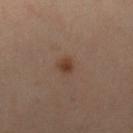This is a cross-polarized tile. About 2 mm across. A female subject aged approximately 40. A close-up tile cropped from a whole-body skin photograph, about 15 mm across. On the right lower leg. The lesion-visualizer software estimated a border-irregularity rating of about 2/10 and radial color variation of about 0.5.